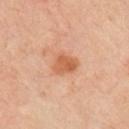The lesion was photographed on a routine skin check and not biopsied; there is no pathology result. The recorded lesion diameter is about 3 mm. The lesion is located on the right upper arm. A close-up tile cropped from a whole-body skin photograph, about 15 mm across. A male patient, approximately 65 years of age. An algorithmic analysis of the crop reported a lesion color around L≈58 a*≈25 b*≈36 in CIELAB, a lesion–skin lightness drop of about 10, and a normalized border contrast of about 7.5. The analysis additionally found a classifier nevus-likeness of about 55/100 and a detector confidence of about 100 out of 100 that the crop contains a lesion. Imaged with cross-polarized lighting.